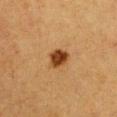Captured during whole-body skin photography for melanoma surveillance; the lesion was not biopsied.